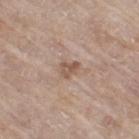Q: Was this lesion biopsied?
A: total-body-photography surveillance lesion; no biopsy
Q: Who is the patient?
A: female, aged 73–77
Q: Lesion location?
A: the leg
Q: How was this image acquired?
A: ~15 mm crop, total-body skin-cancer survey
Q: Automated lesion metrics?
A: a mean CIELAB color near L≈54 a*≈17 b*≈27, a lesion–skin lightness drop of about 9, and a normalized lesion–skin contrast near 7; lesion-presence confidence of about 100/100
Q: What lighting was used for the tile?
A: white-light
Q: What is the lesion's diameter?
A: ≈2.5 mm